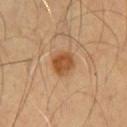Clinical impression:
Captured during whole-body skin photography for melanoma surveillance; the lesion was not biopsied.
Clinical summary:
On the front of the torso. Approximately 3 mm at its widest. The tile uses cross-polarized illumination. A male patient, aged approximately 55. Automated image analysis of the tile measured a footprint of about 7.5 mm², a shape eccentricity near 0.3, and a symmetry-axis asymmetry near 0.2. And it measured a nevus-likeness score of about 100/100 and lesion-presence confidence of about 100/100. A region of skin cropped from a whole-body photographic capture, roughly 15 mm wide.